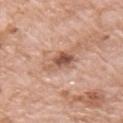Captured during whole-body skin photography for melanoma surveillance; the lesion was not biopsied. The lesion is on the right upper arm. The tile uses white-light illumination. The lesion's longest dimension is about 3.5 mm. Automated image analysis of the tile measured a mean CIELAB color near L≈55 a*≈21 b*≈30, about 12 CIELAB-L* units darker than the surrounding skin, and a normalized lesion–skin contrast near 8. The analysis additionally found border irregularity of about 3.5 on a 0–10 scale, internal color variation of about 6 on a 0–10 scale, and peripheral color asymmetry of about 2. A lesion tile, about 15 mm wide, cut from a 3D total-body photograph. A female patient, roughly 75 years of age.